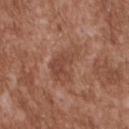Clinical summary:
Imaged with white-light lighting. A 15 mm close-up extracted from a 3D total-body photography capture. The recorded lesion diameter is about 5 mm. A male subject roughly 45 years of age. On the upper back.